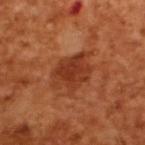Clinical impression: Recorded during total-body skin imaging; not selected for excision or biopsy. Clinical summary: A male patient aged around 65. The tile uses cross-polarized illumination. About 5.5 mm across. A region of skin cropped from a whole-body photographic capture, roughly 15 mm wide. An algorithmic analysis of the crop reported a footprint of about 13 mm² and an outline eccentricity of about 0.7 (0 = round, 1 = elongated). And it measured an automated nevus-likeness rating near 75 out of 100 and a detector confidence of about 100 out of 100 that the crop contains a lesion.